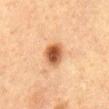{
  "automated_metrics": {
    "area_mm2_approx": 7.5,
    "eccentricity": 0.7,
    "shape_asymmetry": 0.15,
    "cielab_L": 46,
    "cielab_a": 21,
    "cielab_b": 33,
    "vs_skin_darker_L": 15.0,
    "border_irregularity_0_10": 1.5,
    "color_variation_0_10": 5.5,
    "peripheral_color_asymmetry": 1.5,
    "lesion_detection_confidence_0_100": 100
  },
  "image": {
    "source": "total-body photography crop",
    "field_of_view_mm": 15
  },
  "patient": {
    "sex": "male",
    "age_approx": 75
  },
  "site": "abdomen",
  "lesion_size": {
    "long_diameter_mm_approx": 3.5
  },
  "lighting": "cross-polarized"
}